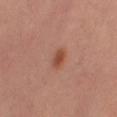<lesion>
  <biopsy_status>not biopsied; imaged during a skin examination</biopsy_status>
  <site>right thigh</site>
  <lesion_size>
    <long_diameter_mm_approx>2.5</long_diameter_mm_approx>
  </lesion_size>
  <patient>
    <sex>female</sex>
    <age_approx>40</age_approx>
  </patient>
  <lighting>cross-polarized</lighting>
  <image>
    <source>total-body photography crop</source>
    <field_of_view_mm>15</field_of_view_mm>
  </image>
</lesion>